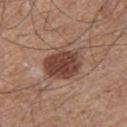No biopsy was performed on this lesion — it was imaged during a full skin examination and was not determined to be concerning. The patient is a male aged approximately 65. The total-body-photography lesion software estimated an average lesion color of about L≈43 a*≈21 b*≈25 (CIELAB), a lesion–skin lightness drop of about 14, and a lesion-to-skin contrast of about 10.5 (normalized; higher = more distinct). Approximately 5.5 mm at its widest. The lesion is on the left thigh. A region of skin cropped from a whole-body photographic capture, roughly 15 mm wide. Imaged with white-light lighting.